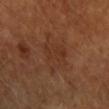| field | value |
|---|---|
| biopsy status | catalogued during a skin exam; not biopsied |